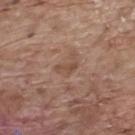Impression:
The lesion was photographed on a routine skin check and not biopsied; there is no pathology result.
Context:
From the mid back. A male subject approximately 70 years of age. This is a white-light tile. A region of skin cropped from a whole-body photographic capture, roughly 15 mm wide.